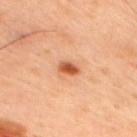{
  "biopsy_status": "not biopsied; imaged during a skin examination",
  "patient": {
    "sex": "male",
    "age_approx": 45
  },
  "site": "right upper arm",
  "lighting": "cross-polarized",
  "image": {
    "source": "total-body photography crop",
    "field_of_view_mm": 15
  },
  "automated_metrics": {
    "area_mm2_approx": 3.5,
    "shape_asymmetry": 0.25,
    "cielab_L": 54,
    "cielab_a": 29,
    "cielab_b": 38,
    "vs_skin_darker_L": 14.0,
    "border_irregularity_0_10": 2.0,
    "color_variation_0_10": 4.0,
    "peripheral_color_asymmetry": 1.0,
    "lesion_detection_confidence_0_100": 100
  }
}